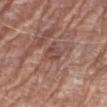This lesion was catalogued during total-body skin photography and was not selected for biopsy. The subject is a male aged 78 to 82. From the right forearm. Cropped from a whole-body photographic skin survey; the tile spans about 15 mm.